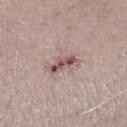Recorded during total-body skin imaging; not selected for excision or biopsy. Measured at roughly 4 mm in maximum diameter. Located on the left lower leg. Imaged with white-light lighting. The subject is a male approximately 65 years of age. A 15 mm crop from a total-body photograph taken for skin-cancer surveillance.